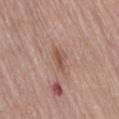workup = imaged on a skin check; not biopsied | location = the right thigh | image = ~15 mm tile from a whole-body skin photo | patient = female, aged approximately 75 | tile lighting = white-light illumination | size = about 3 mm.